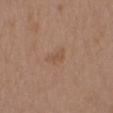Findings:
* site — the chest
* subject — male, aged approximately 40
* lesion size — ≈2.5 mm
* image-analysis metrics — a border-irregularity index near 4/10, internal color variation of about 1 on a 0–10 scale, and a peripheral color-asymmetry measure near 0.5
* image — ~15 mm tile from a whole-body skin photo
* illumination — white-light illumination